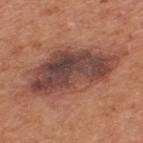<record>
<image>
  <source>total-body photography crop</source>
  <field_of_view_mm>15</field_of_view_mm>
</image>
<lighting>white-light</lighting>
<site>upper back</site>
<lesion_size>
  <long_diameter_mm_approx>12.0</long_diameter_mm_approx>
</lesion_size>
<patient>
  <sex>male</sex>
  <age_approx>65</age_approx>
</patient>
</record>